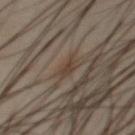workup: imaged on a skin check; not biopsied
body site: the chest
lighting: cross-polarized
image: ~15 mm crop, total-body skin-cancer survey
image-analysis metrics: a lesion area of about 4 mm², an outline eccentricity of about 0.8 (0 = round, 1 = elongated), and a symmetry-axis asymmetry near 0.35
lesion size: ≈3 mm
patient: male, about 45 years old